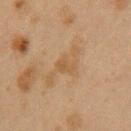workup: total-body-photography surveillance lesion; no biopsy
image source: ~15 mm crop, total-body skin-cancer survey
patient: female, roughly 40 years of age
size: ≈5.5 mm
site: the left upper arm